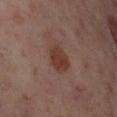workup: total-body-photography surveillance lesion; no biopsy
imaging modality: ~15 mm crop, total-body skin-cancer survey
location: the right lower leg
image-analysis metrics: an area of roughly 7.5 mm², an outline eccentricity of about 0.75 (0 = round, 1 = elongated), and a shape-asymmetry score of about 0.15 (0 = symmetric); a mean CIELAB color near L≈37 a*≈20 b*≈24, roughly 9 lightness units darker than nearby skin, and a normalized lesion–skin contrast near 8; a within-lesion color-variation index near 2/10 and radial color variation of about 0.5
subject: female, aged around 55